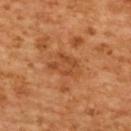{"biopsy_status": "not biopsied; imaged during a skin examination", "image": {"source": "total-body photography crop", "field_of_view_mm": 15}, "site": "upper back", "patient": {"sex": "female", "age_approx": 55}, "automated_metrics": {"area_mm2_approx": 8.0, "shape_asymmetry": 0.3, "cielab_L": 50, "cielab_a": 27, "cielab_b": 41, "vs_skin_darker_L": 8.0, "vs_skin_contrast_norm": 5.5, "border_irregularity_0_10": 3.0, "color_variation_0_10": 2.5, "peripheral_color_asymmetry": 1.0}}